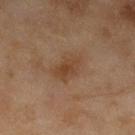Q: What kind of image is this?
A: ~15 mm tile from a whole-body skin photo
Q: Where on the body is the lesion?
A: the left lower leg
Q: What lighting was used for the tile?
A: cross-polarized illumination
Q: Who is the patient?
A: female, roughly 60 years of age